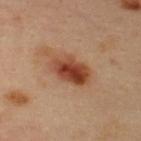A 15 mm crop from a total-body photograph taken for skin-cancer surveillance. The lesion is located on the upper back. A male patient in their mid- to late 30s.